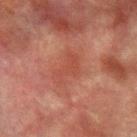biopsy status = no biopsy performed (imaged during a skin exam) | lighting = cross-polarized | patient = male, aged 73 to 77 | site = the left forearm | image source = ~15 mm tile from a whole-body skin photo | size = ~4 mm (longest diameter).